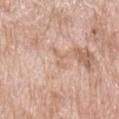Captured during whole-body skin photography for melanoma surveillance; the lesion was not biopsied. A 15 mm crop from a total-body photograph taken for skin-cancer surveillance. A female patient, about 70 years old. On the arm.